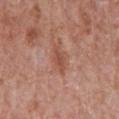Imaged during a routine full-body skin examination; the lesion was not biopsied and no histopathology is available.